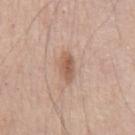follow-up: no biopsy performed (imaged during a skin exam) | image source: ~15 mm tile from a whole-body skin photo | subject: male, aged 53 to 57 | diameter: about 3.5 mm | location: the front of the torso | automated metrics: a lesion color around L≈57 a*≈20 b*≈29 in CIELAB, roughly 11 lightness units darker than nearby skin, and a normalized lesion–skin contrast near 7.5; a nevus-likeness score of about 90/100.